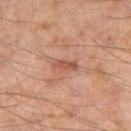Assessment: Recorded during total-body skin imaging; not selected for excision or biopsy. Image and clinical context: A 15 mm close-up tile from a total-body photography series done for melanoma screening. A male subject about 65 years old. An algorithmic analysis of the crop reported an area of roughly 3 mm², a shape eccentricity near 0.95, and two-axis asymmetry of about 0.2. It also reported a border-irregularity rating of about 3/10, a color-variation rating of about 0/10, and a peripheral color-asymmetry measure near 0. Approximately 3 mm at its widest. From the right thigh.